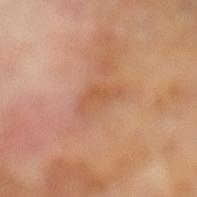automated_metrics:
  area_mm2_approx: 6.5
  eccentricity: 0.85
  shape_asymmetry: 0.45
  cielab_L: 56
  cielab_a: 23
  cielab_b: 36
  vs_skin_darker_L: 6.0
  vs_skin_contrast_norm: 5.0
  border_irregularity_0_10: 4.5
  color_variation_0_10: 2.5
  peripheral_color_asymmetry: 0.5
  nevus_likeness_0_100: 0
  lesion_detection_confidence_0_100: 100
site: left lower leg
image:
  source: total-body photography crop
  field_of_view_mm: 15
patient:
  sex: male
  age_approx: 65
lighting: cross-polarized
lesion_size:
  long_diameter_mm_approx: 4.0